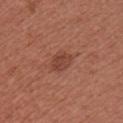  biopsy_status: not biopsied; imaged during a skin examination
  site: chest
  image:
    source: total-body photography crop
    field_of_view_mm: 15
  patient:
    sex: female
    age_approx: 25